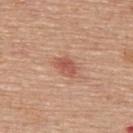Notes:
* workup: imaged on a skin check; not biopsied